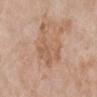A female subject, about 70 years old. The lesion is on the left lower leg. A close-up tile cropped from a whole-body skin photograph, about 15 mm across. The recorded lesion diameter is about 5.5 mm. Imaged with white-light lighting. The total-body-photography lesion software estimated an area of roughly 14 mm², a shape eccentricity near 0.65, and two-axis asymmetry of about 0.45. The software also gave an automated nevus-likeness rating near 0 out of 100.